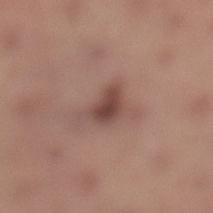  biopsy_status: not biopsied; imaged during a skin examination
  site: left lower leg
  patient:
    sex: female
    age_approx: 65
  image:
    source: total-body photography crop
    field_of_view_mm: 15
  lesion_size:
    long_diameter_mm_approx: 4.0
  lighting: white-light
  automated_metrics:
    area_mm2_approx: 6.5
    eccentricity: 0.75
    shape_asymmetry: 0.4